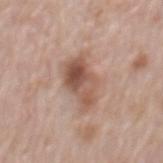notes: imaged on a skin check; not biopsied | patient: male, roughly 70 years of age | anatomic site: the lower back | image-analysis metrics: a mean CIELAB color near L≈54 a*≈19 b*≈27; a classifier nevus-likeness of about 70/100 and a detector confidence of about 100 out of 100 that the crop contains a lesion | imaging modality: ~15 mm tile from a whole-body skin photo.